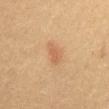Q: Was a biopsy performed?
A: total-body-photography surveillance lesion; no biopsy
Q: Illumination type?
A: cross-polarized illumination
Q: Who is the patient?
A: female, aged approximately 50
Q: How was this image acquired?
A: ~15 mm crop, total-body skin-cancer survey
Q: Lesion location?
A: the mid back